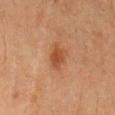notes: imaged on a skin check; not biopsied | lighting: cross-polarized | patient: male, approximately 65 years of age | lesion diameter: about 3.5 mm | automated metrics: a lesion color around L≈40 a*≈22 b*≈31 in CIELAB and a normalized lesion–skin contrast near 7.5; internal color variation of about 2 on a 0–10 scale and radial color variation of about 0.5; a detector confidence of about 100 out of 100 that the crop contains a lesion | site: the back | image: ~15 mm tile from a whole-body skin photo.